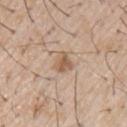workup: catalogued during a skin exam; not biopsied | automated lesion analysis: a lesion-to-skin contrast of about 7 (normalized; higher = more distinct); internal color variation of about 3 on a 0–10 scale and radial color variation of about 1; an automated nevus-likeness rating near 85 out of 100 and a lesion-detection confidence of about 100/100 | subject: male, in their mid-50s | imaging modality: ~15 mm tile from a whole-body skin photo | lesion size: ≈2.5 mm | lighting: white-light illumination.